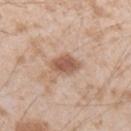Findings:
* follow-up — total-body-photography surveillance lesion; no biopsy
* site — the left forearm
* acquisition — 15 mm crop, total-body photography
* lesion size — ≈3 mm
* lighting — white-light illumination
* subject — male, in their mid- to late 20s